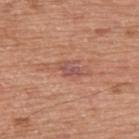Part of a total-body skin-imaging series; this lesion was reviewed on a skin check and was not flagged for biopsy. A male patient, aged 73 to 77. The recorded lesion diameter is about 3 mm. Located on the back. This image is a 15 mm lesion crop taken from a total-body photograph. The total-body-photography lesion software estimated an eccentricity of roughly 0.85 and a shape-asymmetry score of about 0.3 (0 = symmetric). The analysis additionally found a classifier nevus-likeness of about 0/100.